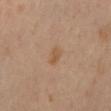This lesion was catalogued during total-body skin photography and was not selected for biopsy. From the back. Measured at roughly 2.5 mm in maximum diameter. Automated tile analysis of the lesion measured an area of roughly 3 mm², a shape eccentricity near 0.85, and a shape-asymmetry score of about 0.3 (0 = symmetric). The tile uses cross-polarized illumination. A female patient in their mid-50s. A 15 mm close-up extracted from a 3D total-body photography capture.